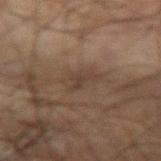notes = imaged on a skin check; not biopsied | lighting = cross-polarized | site = the left forearm | patient = male, aged approximately 70 | acquisition = 15 mm crop, total-body photography | diameter = ≈3.5 mm.